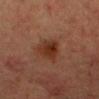Recorded during total-body skin imaging; not selected for excision or biopsy.
The recorded lesion diameter is about 4 mm.
A 15 mm close-up extracted from a 3D total-body photography capture.
The total-body-photography lesion software estimated a lesion area of about 8 mm², an eccentricity of roughly 0.7, and two-axis asymmetry of about 0.25. The software also gave an average lesion color of about L≈30 a*≈22 b*≈28 (CIELAB), roughly 8 lightness units darker than nearby skin, and a normalized lesion–skin contrast near 9.
On the head or neck.
A male subject, aged 33 to 37.